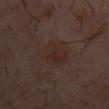Assessment:
Part of a total-body skin-imaging series; this lesion was reviewed on a skin check and was not flagged for biopsy.
Acquisition and patient details:
On the mid back. The patient is a male aged approximately 60. Imaged with cross-polarized lighting. A lesion tile, about 15 mm wide, cut from a 3D total-body photograph.